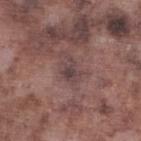Imaged during a routine full-body skin examination; the lesion was not biopsied and no histopathology is available. A close-up tile cropped from a whole-body skin photograph, about 15 mm across. From the left lower leg. A male subject, aged approximately 75. An algorithmic analysis of the crop reported an area of roughly 5.5 mm², an eccentricity of roughly 0.8, and a symmetry-axis asymmetry near 0.4. This is a white-light tile.